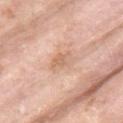The lesion was photographed on a routine skin check and not biopsied; there is no pathology result. Cropped from a whole-body photographic skin survey; the tile spans about 15 mm. The lesion is on the upper back. The patient is a female roughly 70 years of age. Captured under white-light illumination.